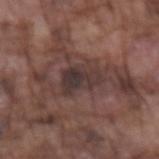Clinical summary:
The subject is a male aged 73–77. From the left forearm. Longest diameter approximately 4.5 mm. The total-body-photography lesion software estimated roughly 8 lightness units darker than nearby skin and a normalized border contrast of about 8.5. The analysis additionally found a border-irregularity rating of about 4.5/10. The analysis additionally found an automated nevus-likeness rating near 0 out of 100. A roughly 15 mm field-of-view crop from a total-body skin photograph.